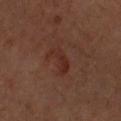– biopsy status · no biopsy performed (imaged during a skin exam)
– diameter · ~3.5 mm (longest diameter)
– image source · ~15 mm crop, total-body skin-cancer survey
– subject · male, aged 63–67
– body site · the right upper arm